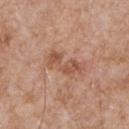Clinical impression:
This lesion was catalogued during total-body skin photography and was not selected for biopsy.
Background:
Longest diameter approximately 4.5 mm. On the front of the torso. This is a white-light tile. A male subject, approximately 65 years of age. A lesion tile, about 15 mm wide, cut from a 3D total-body photograph.